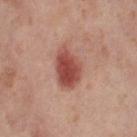Part of a total-body skin-imaging series; this lesion was reviewed on a skin check and was not flagged for biopsy. A female patient, approximately 55 years of age. From the left thigh. Imaged with cross-polarized lighting. Cropped from a whole-body photographic skin survey; the tile spans about 15 mm. The recorded lesion diameter is about 5 mm.